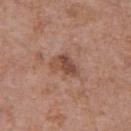Clinical impression:
Imaged during a routine full-body skin examination; the lesion was not biopsied and no histopathology is available.
Acquisition and patient details:
The recorded lesion diameter is about 3.5 mm. Located on the chest. A 15 mm close-up extracted from a 3D total-body photography capture. A male subject about 70 years old. Automated image analysis of the tile measured a lesion area of about 6.5 mm², an outline eccentricity of about 0.75 (0 = round, 1 = elongated), and a symmetry-axis asymmetry near 0.4. The analysis additionally found a normalized border contrast of about 7.5. And it measured a classifier nevus-likeness of about 5/100 and lesion-presence confidence of about 100/100. Imaged with white-light lighting.